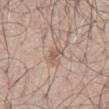• notes — no biopsy performed (imaged during a skin exam)
• lesion size — ≈2 mm
• tile lighting — white-light illumination
• body site — the front of the torso
• image — ~15 mm tile from a whole-body skin photo
• patient — male, aged approximately 55